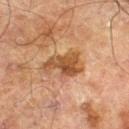{
  "biopsy_status": "not biopsied; imaged during a skin examination",
  "lighting": "cross-polarized",
  "site": "left thigh",
  "image": {
    "source": "total-body photography crop",
    "field_of_view_mm": 15
  },
  "patient": {
    "sex": "male",
    "age_approx": 70
  },
  "lesion_size": {
    "long_diameter_mm_approx": 4.5
  }
}